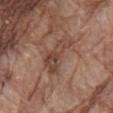Notes:
– image source — ~15 mm tile from a whole-body skin photo
– body site — the mid back
– illumination — white-light
– subject — male, aged 78–82
– lesion diameter — about 5.5 mm
– TBP lesion metrics — a border-irregularity index near 5/10